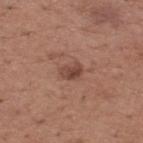Captured during whole-body skin photography for melanoma surveillance; the lesion was not biopsied.
A male patient, roughly 65 years of age.
Captured under white-light illumination.
Measured at roughly 2.5 mm in maximum diameter.
Cropped from a total-body skin-imaging series; the visible field is about 15 mm.
The total-body-photography lesion software estimated a within-lesion color-variation index near 4.5/10 and peripheral color asymmetry of about 1.5.
The lesion is on the upper back.